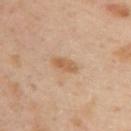Q: Was a biopsy performed?
A: imaged on a skin check; not biopsied
Q: Lesion size?
A: about 3 mm
Q: Patient demographics?
A: female, aged around 40
Q: What kind of image is this?
A: ~15 mm tile from a whole-body skin photo
Q: Automated lesion metrics?
A: a footprint of about 4 mm², a shape eccentricity near 0.9, and a symmetry-axis asymmetry near 0.2; an automated nevus-likeness rating near 15 out of 100 and lesion-presence confidence of about 100/100
Q: What lighting was used for the tile?
A: cross-polarized illumination
Q: What is the anatomic site?
A: the upper back